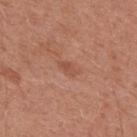The lesion was photographed on a routine skin check and not biopsied; there is no pathology result. Automated tile analysis of the lesion measured a lesion area of about 2.5 mm², an outline eccentricity of about 0.85 (0 = round, 1 = elongated), and a symmetry-axis asymmetry near 0.35. It also reported border irregularity of about 3.5 on a 0–10 scale and peripheral color asymmetry of about 0.5. A region of skin cropped from a whole-body photographic capture, roughly 15 mm wide. The lesion is on the upper back. A male subject approximately 55 years of age. Measured at roughly 2.5 mm in maximum diameter. Captured under white-light illumination.